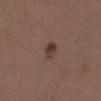biopsy status: no biopsy performed (imaged during a skin exam) | image-analysis metrics: an area of roughly 4 mm², an outline eccentricity of about 0.75 (0 = round, 1 = elongated), and a symmetry-axis asymmetry near 0.2; a mean CIELAB color near L≈37 a*≈18 b*≈24, a lesion–skin lightness drop of about 9, and a lesion-to-skin contrast of about 8 (normalized; higher = more distinct); a classifier nevus-likeness of about 95/100 | body site: the left lower leg | lesion diameter: ≈2.5 mm | imaging modality: ~15 mm tile from a whole-body skin photo | subject: female, aged around 40.